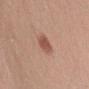- workup · no biopsy performed (imaged during a skin exam)
- patient · male, in their mid- to late 50s
- image-analysis metrics · a border-irregularity index near 2/10
- lesion diameter · ~3 mm (longest diameter)
- imaging modality · ~15 mm tile from a whole-body skin photo
- lighting · white-light illumination
- site · the right upper arm